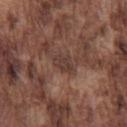biopsy status: imaged on a skin check; not biopsied | lesion diameter: about 2.5 mm | acquisition: total-body-photography crop, ~15 mm field of view | subject: male, aged approximately 75 | lighting: white-light illumination | automated lesion analysis: a mean CIELAB color near L≈37 a*≈18 b*≈22, about 7 CIELAB-L* units darker than the surrounding skin, and a normalized border contrast of about 6.5; a border-irregularity index near 2.5/10, internal color variation of about 2 on a 0–10 scale, and radial color variation of about 0.5 | body site: the chest.